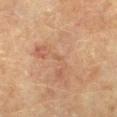Clinical impression: Part of a total-body skin-imaging series; this lesion was reviewed on a skin check and was not flagged for biopsy. Clinical summary: A 15 mm crop from a total-body photograph taken for skin-cancer surveillance. The patient is a female approximately 80 years of age. Automated tile analysis of the lesion measured a within-lesion color-variation index near 0/10 and a peripheral color-asymmetry measure near 0. The analysis additionally found a nevus-likeness score of about 0/100 and a detector confidence of about 90 out of 100 that the crop contains a lesion. Approximately 1.5 mm at its widest. The tile uses cross-polarized illumination. From the right forearm.